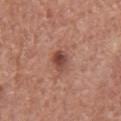Part of a total-body skin-imaging series; this lesion was reviewed on a skin check and was not flagged for biopsy. Approximately 3 mm at its widest. The subject is a male about 75 years old. A 15 mm close-up tile from a total-body photography series done for melanoma screening. An algorithmic analysis of the crop reported a lesion area of about 4.5 mm² and an eccentricity of roughly 0.8. And it measured a border-irregularity index near 2.5/10 and internal color variation of about 5.5 on a 0–10 scale. Located on the chest. Captured under white-light illumination.